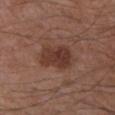Notes:
- biopsy status — total-body-photography surveillance lesion; no biopsy
- image source — ~15 mm tile from a whole-body skin photo
- TBP lesion metrics — a lesion color around L≈37 a*≈20 b*≈25 in CIELAB, roughly 10 lightness units darker than nearby skin, and a lesion-to-skin contrast of about 8.5 (normalized; higher = more distinct)
- diameter — about 4.5 mm
- illumination — white-light illumination
- patient — male, roughly 50 years of age
- anatomic site — the left upper arm